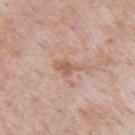Notes:
* follow-up — catalogued during a skin exam; not biopsied
* image source — 15 mm crop, total-body photography
* diameter — ~3.5 mm (longest diameter)
* TBP lesion metrics — an area of roughly 4.5 mm², an eccentricity of roughly 0.9, and a shape-asymmetry score of about 0.4 (0 = symmetric); a border-irregularity rating of about 4.5/10, a within-lesion color-variation index near 1.5/10, and radial color variation of about 0.5; a nevus-likeness score of about 0/100 and a detector confidence of about 100 out of 100 that the crop contains a lesion
* subject — male, aged around 55
* site — the arm